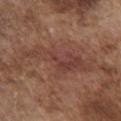biopsy status: total-body-photography surveillance lesion; no biopsy | image: 15 mm crop, total-body photography | anatomic site: the chest | automated lesion analysis: a lesion area of about 18 mm², an outline eccentricity of about 0.95 (0 = round, 1 = elongated), and a shape-asymmetry score of about 0.45 (0 = symmetric); a mean CIELAB color near L≈41 a*≈22 b*≈25 and a normalized lesion–skin contrast near 6; a border-irregularity index near 8/10 and a color-variation rating of about 4.5/10; a lesion-detection confidence of about 80/100 | diameter: about 8.5 mm | patient: male, in their mid-70s.